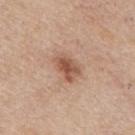Clinical impression: Captured during whole-body skin photography for melanoma surveillance; the lesion was not biopsied. Context: A 15 mm crop from a total-body photograph taken for skin-cancer surveillance. Captured under white-light illumination. The lesion is located on the mid back. An algorithmic analysis of the crop reported a lesion color around L≈54 a*≈21 b*≈30 in CIELAB, about 12 CIELAB-L* units darker than the surrounding skin, and a normalized border contrast of about 8. The analysis additionally found a border-irregularity rating of about 2.5/10, a within-lesion color-variation index near 4.5/10, and radial color variation of about 1.5. It also reported a nevus-likeness score of about 80/100 and a detector confidence of about 100 out of 100 that the crop contains a lesion. Longest diameter approximately 3.5 mm. A male patient, in their mid- to late 50s.